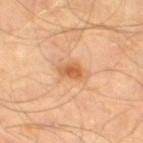Part of a total-body skin-imaging series; this lesion was reviewed on a skin check and was not flagged for biopsy. Imaged with cross-polarized lighting. The lesion is located on the right leg. The recorded lesion diameter is about 3 mm. Cropped from a total-body skin-imaging series; the visible field is about 15 mm. A male subject aged 53 to 57. Automated tile analysis of the lesion measured a nevus-likeness score of about 80/100 and lesion-presence confidence of about 100/100.